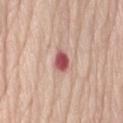subject: female, approximately 75 years of age; site: the lower back; image source: ~15 mm crop, total-body skin-cancer survey.